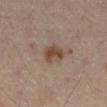No biopsy was performed on this lesion — it was imaged during a full skin examination and was not determined to be concerning.
A male subject, about 50 years old.
A close-up tile cropped from a whole-body skin photograph, about 15 mm across.
Located on the left leg.
The lesion-visualizer software estimated a lesion area of about 5.5 mm² and a symmetry-axis asymmetry near 0.3. It also reported a border-irregularity rating of about 3/10, a color-variation rating of about 2.5/10, and a peripheral color-asymmetry measure near 1.